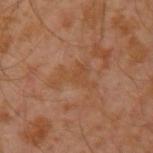acquisition: total-body-photography crop, ~15 mm field of view | patient: male, aged around 30 | site: the arm | lighting: cross-polarized | automated metrics: border irregularity of about 8.5 on a 0–10 scale, a color-variation rating of about 1.5/10, and radial color variation of about 0.5; a lesion-detection confidence of about 100/100.